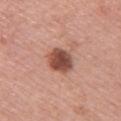{
  "biopsy_status": "not biopsied; imaged during a skin examination",
  "site": "upper back",
  "lighting": "white-light",
  "image": {
    "source": "total-body photography crop",
    "field_of_view_mm": 15
  },
  "patient": {
    "sex": "female",
    "age_approx": 60
  },
  "automated_metrics": {
    "area_mm2_approx": 8.5,
    "eccentricity": 0.65,
    "shape_asymmetry": 0.15
  },
  "lesion_size": {
    "long_diameter_mm_approx": 3.5
  }
}